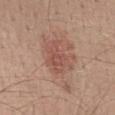- follow-up · total-body-photography surveillance lesion; no biopsy
- patient · male, aged 38–42
- body site · the mid back
- lighting · white-light illumination
- automated metrics · a color-variation rating of about 3/10 and peripheral color asymmetry of about 1; a classifier nevus-likeness of about 5/100 and a lesion-detection confidence of about 100/100
- size · ≈4 mm
- acquisition · 15 mm crop, total-body photography